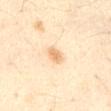Q: Was this lesion biopsied?
A: imaged on a skin check; not biopsied
Q: What is the anatomic site?
A: the chest
Q: What is the imaging modality?
A: total-body-photography crop, ~15 mm field of view
Q: What lighting was used for the tile?
A: cross-polarized illumination
Q: Lesion size?
A: ≈2.5 mm
Q: What are the patient's age and sex?
A: male, aged 48–52